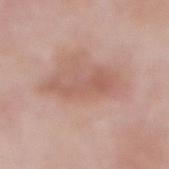follow-up=total-body-photography surveillance lesion; no biopsy | subject=male, approximately 55 years of age | anatomic site=the abdomen | image=total-body-photography crop, ~15 mm field of view.